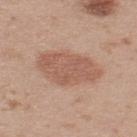| feature | finding |
|---|---|
| workup | total-body-photography surveillance lesion; no biopsy |
| subject | male, aged 33–37 |
| anatomic site | the upper back |
| image-analysis metrics | a lesion area of about 20 mm², an eccentricity of roughly 0.8, and a shape-asymmetry score of about 0.15 (0 = symmetric); an average lesion color of about L≈57 a*≈21 b*≈28 (CIELAB), roughly 9 lightness units darker than nearby skin, and a normalized lesion–skin contrast near 6.5; a border-irregularity index near 2/10, a color-variation rating of about 2.5/10, and a peripheral color-asymmetry measure near 1 |
| lighting | white-light illumination |
| lesion diameter | ~6.5 mm (longest diameter) |
| image | ~15 mm tile from a whole-body skin photo |